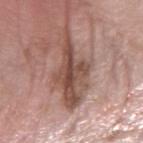This lesion was catalogued during total-body skin photography and was not selected for biopsy. The lesion is located on the right forearm. A roughly 15 mm field-of-view crop from a total-body skin photograph. An algorithmic analysis of the crop reported a lesion–skin lightness drop of about 13 and a normalized border contrast of about 9. The software also gave radial color variation of about 2. And it measured a detector confidence of about 85 out of 100 that the crop contains a lesion. The lesion's longest dimension is about 9.5 mm. A female subject, aged 73 to 77.